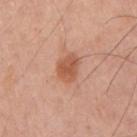No biopsy was performed on this lesion — it was imaged during a full skin examination and was not determined to be concerning. This is a white-light tile. The lesion is on the right upper arm. Approximately 3 mm at its widest. The subject is a male aged 53 to 57. The lesion-visualizer software estimated a normalized lesion–skin contrast near 7.5. The analysis additionally found a border-irregularity index near 2/10. A 15 mm crop from a total-body photograph taken for skin-cancer surveillance.